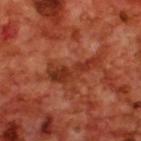notes = imaged on a skin check; not biopsied
lesion size = ~6 mm (longest diameter)
site = the upper back
image source = ~15 mm tile from a whole-body skin photo
lighting = cross-polarized illumination
patient = male, aged 68–72